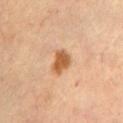image: ~15 mm tile from a whole-body skin photo; diameter: ~3 mm (longest diameter); patient: female, roughly 60 years of age; location: the chest; automated metrics: a nevus-likeness score of about 95/100 and a detector confidence of about 100 out of 100 that the crop contains a lesion; tile lighting: cross-polarized illumination.